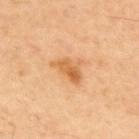No biopsy was performed on this lesion — it was imaged during a full skin examination and was not determined to be concerning. Cropped from a total-body skin-imaging series; the visible field is about 15 mm. From the upper back. A male patient, in their 60s.